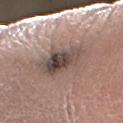Assessment:
Recorded during total-body skin imaging; not selected for excision or biopsy.
Clinical summary:
A male patient roughly 55 years of age. The lesion is on the left forearm. Cropped from a total-body skin-imaging series; the visible field is about 15 mm. Longest diameter approximately 6 mm. This is a white-light tile.